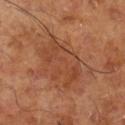Q: Who is the patient?
A: male, in their mid-60s
Q: What kind of image is this?
A: 15 mm crop, total-body photography
Q: How large is the lesion?
A: about 6 mm
Q: Illumination type?
A: cross-polarized
Q: What is the anatomic site?
A: the right lower leg